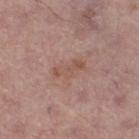Recorded during total-body skin imaging; not selected for excision or biopsy.
A male subject aged 53–57.
From the right thigh.
A 15 mm close-up tile from a total-body photography series done for melanoma screening.